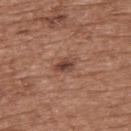biopsy status — total-body-photography surveillance lesion; no biopsy | location — the back | subject — male, about 75 years old | image source — ~15 mm crop, total-body skin-cancer survey.